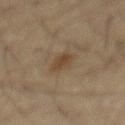A 15 mm close-up extracted from a 3D total-body photography capture.
A male subject roughly 60 years of age.